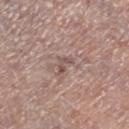Recorded during total-body skin imaging; not selected for excision or biopsy. From the right lower leg. Automated image analysis of the tile measured a lesion area of about 2.5 mm², an eccentricity of roughly 0.9, and a symmetry-axis asymmetry near 0.6. The analysis additionally found a border-irregularity rating of about 6.5/10, internal color variation of about 0 on a 0–10 scale, and a peripheral color-asymmetry measure near 0. The tile uses white-light illumination. The subject is a male in their mid-50s. Cropped from a total-body skin-imaging series; the visible field is about 15 mm. Approximately 2.5 mm at its widest.